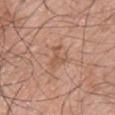Case summary:
– follow-up — no biopsy performed (imaged during a skin exam)
– lighting — white-light
– anatomic site — the chest
– diameter — ≈3 mm
– subject — male, aged approximately 70
– acquisition — ~15 mm crop, total-body skin-cancer survey